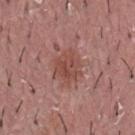workup=total-body-photography surveillance lesion; no biopsy | anatomic site=the chest | image-analysis metrics=an eccentricity of roughly 0.6; a classifier nevus-likeness of about 30/100 and lesion-presence confidence of about 100/100 | patient=male, aged 38 to 42 | imaging modality=total-body-photography crop, ~15 mm field of view | illumination=white-light illumination.